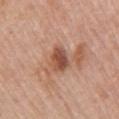Q: Is there a histopathology result?
A: catalogued during a skin exam; not biopsied
Q: Where on the body is the lesion?
A: the right upper arm
Q: What are the patient's age and sex?
A: female, in their mid-60s
Q: Lesion size?
A: about 3 mm
Q: Illumination type?
A: white-light illumination
Q: How was this image acquired?
A: ~15 mm tile from a whole-body skin photo